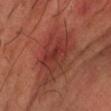workup = no biopsy performed (imaged during a skin exam) | lighting = cross-polarized illumination | location = the left forearm | lesion size = ≈6.5 mm | subject = male, about 60 years old | image source = ~15 mm crop, total-body skin-cancer survey.